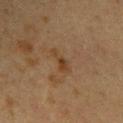Case summary:
– notes — imaged on a skin check; not biopsied
– subject — female, about 40 years old
– diameter — ~3.5 mm (longest diameter)
– acquisition — total-body-photography crop, ~15 mm field of view
– anatomic site — the right upper arm